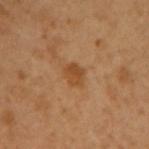No biopsy was performed on this lesion — it was imaged during a full skin examination and was not determined to be concerning.
About 3 mm across.
The lesion is located on the arm.
A 15 mm close-up tile from a total-body photography series done for melanoma screening.
The tile uses cross-polarized illumination.
A male subject, aged 48 to 52.